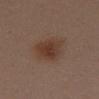Assessment: The lesion was tiled from a total-body skin photograph and was not biopsied. Acquisition and patient details: Captured under white-light illumination. The subject is a female in their 40s. Located on the mid back. A roughly 15 mm field-of-view crop from a total-body skin photograph. An algorithmic analysis of the crop reported a lesion area of about 11 mm², an outline eccentricity of about 0.6 (0 = round, 1 = elongated), and two-axis asymmetry of about 0.2. And it measured peripheral color asymmetry of about 1. About 4.5 mm across.